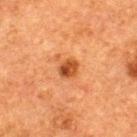Assessment:
Part of a total-body skin-imaging series; this lesion was reviewed on a skin check and was not flagged for biopsy.
Clinical summary:
A roughly 15 mm field-of-view crop from a total-body skin photograph. Longest diameter approximately 2.5 mm. The lesion is located on the upper back. Imaged with cross-polarized lighting. A male subject, approximately 50 years of age. Automated image analysis of the tile measured a mean CIELAB color near L≈40 a*≈25 b*≈36, a lesion–skin lightness drop of about 12, and a normalized border contrast of about 9.5. The analysis additionally found internal color variation of about 5 on a 0–10 scale and a peripheral color-asymmetry measure near 2.